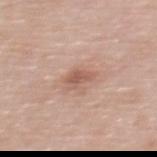Impression:
The lesion was tiled from a total-body skin photograph and was not biopsied.
Context:
On the upper back. The lesion-visualizer software estimated a footprint of about 4 mm², an eccentricity of roughly 0.75, and a symmetry-axis asymmetry near 0.3. And it measured an automated nevus-likeness rating near 20 out of 100 and lesion-presence confidence of about 100/100. Captured under white-light illumination. A male subject aged 58 to 62. This image is a 15 mm lesion crop taken from a total-body photograph. Approximately 2.5 mm at its widest.